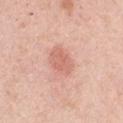biopsy status: total-body-photography surveillance lesion; no biopsy | acquisition: total-body-photography crop, ~15 mm field of view | location: the chest | size: ~3.5 mm (longest diameter) | patient: male, in their 50s | illumination: white-light | automated lesion analysis: an area of roughly 7.5 mm², an eccentricity of roughly 0.75, and a symmetry-axis asymmetry near 0.15; a border-irregularity index near 1.5/10, internal color variation of about 2 on a 0–10 scale, and a peripheral color-asymmetry measure near 0.5; a nevus-likeness score of about 75/100 and lesion-presence confidence of about 100/100.